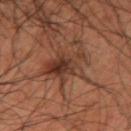Q: Is there a histopathology result?
A: imaged on a skin check; not biopsied
Q: Automated lesion metrics?
A: an area of roughly 14 mm², a shape eccentricity near 0.5, and a shape-asymmetry score of about 0.55 (0 = symmetric); border irregularity of about 9 on a 0–10 scale, a color-variation rating of about 7/10, and peripheral color asymmetry of about 2.5
Q: What is the lesion's diameter?
A: ~6 mm (longest diameter)
Q: How was this image acquired?
A: ~15 mm tile from a whole-body skin photo
Q: What is the anatomic site?
A: the arm
Q: Who is the patient?
A: male, in their 40s